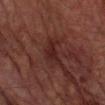| key | value |
|---|---|
| subject | male, about 75 years old |
| image source | ~15 mm crop, total-body skin-cancer survey |
| anatomic site | the left forearm |